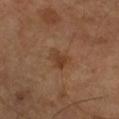The lesion was photographed on a routine skin check and not biopsied; there is no pathology result. Measured at roughly 3 mm in maximum diameter. A 15 mm close-up extracted from a 3D total-body photography capture. The subject is a male in their mid- to late 60s. Imaged with cross-polarized lighting. From the right lower leg.